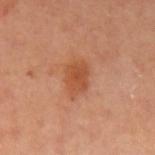A male patient in their 40s. A 15 mm crop from a total-body photograph taken for skin-cancer surveillance. From the left upper arm.